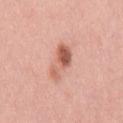{"biopsy_status": "not biopsied; imaged during a skin examination", "site": "mid back", "image": {"source": "total-body photography crop", "field_of_view_mm": 15}, "patient": {"sex": "male", "age_approx": 60}}